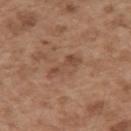Case summary:
• biopsy status — catalogued during a skin exam; not biopsied
• anatomic site — the arm
• acquisition — 15 mm crop, total-body photography
• patient — male, aged approximately 55
• lighting — white-light